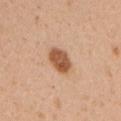follow-up: imaged on a skin check; not biopsied
location: the right upper arm
automated lesion analysis: an area of roughly 7 mm², a shape eccentricity near 0.7, and two-axis asymmetry of about 0.15; a nevus-likeness score of about 100/100
diameter: about 3.5 mm
tile lighting: white-light illumination
imaging modality: 15 mm crop, total-body photography
subject: female, about 30 years old